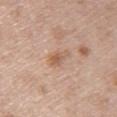  biopsy_status: not biopsied; imaged during a skin examination
  site: arm
  patient:
    sex: female
    age_approx: 50
  image:
    source: total-body photography crop
    field_of_view_mm: 15